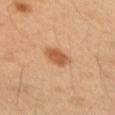| feature | finding |
|---|---|
| body site | the left arm |
| imaging modality | 15 mm crop, total-body photography |
| diameter | ≈3 mm |
| illumination | cross-polarized illumination |
| patient | male, approximately 50 years of age |